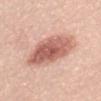follow-up: total-body-photography surveillance lesion; no biopsy
image-analysis metrics: an area of roughly 22 mm², an outline eccentricity of about 0.85 (0 = round, 1 = elongated), and two-axis asymmetry of about 0.1; a mean CIELAB color near L≈61 a*≈25 b*≈28, a lesion–skin lightness drop of about 15, and a normalized border contrast of about 9; border irregularity of about 1.5 on a 0–10 scale and a within-lesion color-variation index near 6/10; a nevus-likeness score of about 90/100 and lesion-presence confidence of about 100/100
illumination: white-light
anatomic site: the front of the torso
acquisition: 15 mm crop, total-body photography
lesion diameter: ≈7.5 mm
subject: female, in their mid- to late 60s